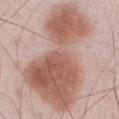Q: Lesion size?
A: ≈13 mm
Q: How was this image acquired?
A: ~15 mm crop, total-body skin-cancer survey
Q: What lighting was used for the tile?
A: white-light
Q: What are the patient's age and sex?
A: male, aged approximately 55
Q: Where on the body is the lesion?
A: the abdomen